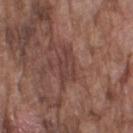A lesion tile, about 15 mm wide, cut from a 3D total-body photograph. A male patient, in their mid- to late 70s. Longest diameter approximately 4 mm. Located on the right upper arm. Captured under white-light illumination.